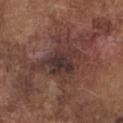Recorded during total-body skin imaging; not selected for excision or biopsy.
Imaged with white-light lighting.
A male patient approximately 75 years of age.
The lesion is located on the chest.
A 15 mm close-up tile from a total-body photography series done for melanoma screening.
About 6 mm across.